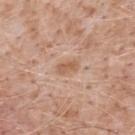<tbp_lesion>
  <biopsy_status>not biopsied; imaged during a skin examination</biopsy_status>
  <site>upper back</site>
  <image>
    <source>total-body photography crop</source>
    <field_of_view_mm>15</field_of_view_mm>
  </image>
  <patient>
    <sex>male</sex>
    <age_approx>50</age_approx>
  </patient>
</tbp_lesion>